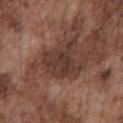Findings:
• notes · catalogued during a skin exam; not biopsied
• imaging modality · ~15 mm crop, total-body skin-cancer survey
• anatomic site · the front of the torso
• patient · male, approximately 75 years of age